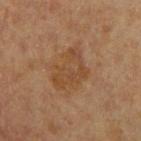Impression:
Imaged during a routine full-body skin examination; the lesion was not biopsied and no histopathology is available.
Clinical summary:
The lesion is on the arm. Approximately 5 mm at its widest. Cropped from a total-body skin-imaging series; the visible field is about 15 mm. A male patient in their mid- to late 60s. Captured under cross-polarized illumination.